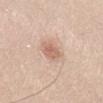Assessment: Recorded during total-body skin imaging; not selected for excision or biopsy. Acquisition and patient details: A 15 mm crop from a total-body photograph taken for skin-cancer surveillance. Imaged with white-light lighting. A male patient, about 30 years old. Automated image analysis of the tile measured a footprint of about 5 mm², an eccentricity of roughly 0.5, and a shape-asymmetry score of about 0.2 (0 = symmetric). The analysis additionally found an automated nevus-likeness rating near 85 out of 100 and a detector confidence of about 100 out of 100 that the crop contains a lesion. On the abdomen.